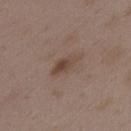notes: catalogued during a skin exam; not biopsied | imaging modality: total-body-photography crop, ~15 mm field of view | anatomic site: the back | subject: female, aged 33–37 | illumination: white-light illumination | TBP lesion metrics: a footprint of about 6 mm², an outline eccentricity of about 0.85 (0 = round, 1 = elongated), and two-axis asymmetry of about 0.25; an average lesion color of about L≈45 a*≈15 b*≈24 (CIELAB), about 7 CIELAB-L* units darker than the surrounding skin, and a normalized border contrast of about 6.5; an automated nevus-likeness rating near 5 out of 100 and a lesion-detection confidence of about 100/100.